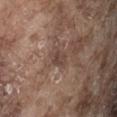Notes:
– workup: catalogued during a skin exam; not biopsied
– size: ~2.5 mm (longest diameter)
– illumination: white-light illumination
– patient: male, approximately 75 years of age
– body site: the abdomen
– image: ~15 mm crop, total-body skin-cancer survey